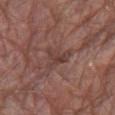notes: total-body-photography surveillance lesion; no biopsy | tile lighting: white-light illumination | TBP lesion metrics: a footprint of about 5.5 mm², a shape eccentricity near 0.75, and a symmetry-axis asymmetry near 0.55; a normalized border contrast of about 6; a border-irregularity index near 6.5/10, a within-lesion color-variation index near 2.5/10, and peripheral color asymmetry of about 0.5; a lesion-detection confidence of about 85/100 | site: the right thigh | lesion size: ≈3.5 mm | acquisition: total-body-photography crop, ~15 mm field of view | patient: male, approximately 80 years of age.